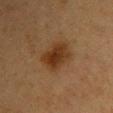Recorded during total-body skin imaging; not selected for excision or biopsy. A female patient, in their mid- to late 40s. This is a cross-polarized tile. Automated image analysis of the tile measured a shape-asymmetry score of about 0.15 (0 = symmetric). The analysis additionally found a border-irregularity rating of about 2/10, a color-variation rating of about 3.5/10, and a peripheral color-asymmetry measure near 1. A 15 mm crop from a total-body photograph taken for skin-cancer surveillance. From the back. Measured at roughly 4 mm in maximum diameter.